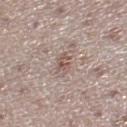• notes — imaged on a skin check; not biopsied
• site — the right lower leg
• subject — female, aged approximately 50
• lesion diameter — ≈2.5 mm
• tile lighting — white-light
• image source — ~15 mm tile from a whole-body skin photo